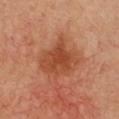biopsy status: imaged on a skin check; not biopsied | TBP lesion metrics: an area of roughly 18 mm² and a shape eccentricity near 0.5; an average lesion color of about L≈49 a*≈29 b*≈37 (CIELAB), roughly 10 lightness units darker than nearby skin, and a normalized border contrast of about 7.5; a color-variation rating of about 4/10 and a peripheral color-asymmetry measure near 1 | image: total-body-photography crop, ~15 mm field of view | illumination: cross-polarized illumination | subject: female, roughly 40 years of age | location: the chest.